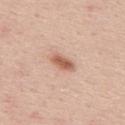The lesion is on the upper back. A 15 mm crop from a total-body photograph taken for skin-cancer surveillance. The patient is a male in their mid-40s. Longest diameter approximately 3 mm. This is a white-light tile.